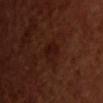Imaged during a routine full-body skin examination; the lesion was not biopsied and no histopathology is available.